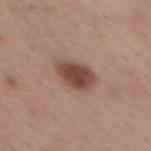Q: Was a biopsy performed?
A: total-body-photography surveillance lesion; no biopsy
Q: How large is the lesion?
A: about 4.5 mm
Q: Patient demographics?
A: female, about 50 years old
Q: What kind of image is this?
A: ~15 mm crop, total-body skin-cancer survey
Q: Automated lesion metrics?
A: a lesion area of about 9.5 mm², an eccentricity of roughly 0.8, and a symmetry-axis asymmetry near 0.25; a lesion color around L≈46 a*≈21 b*≈25 in CIELAB, a lesion–skin lightness drop of about 14, and a normalized lesion–skin contrast near 10.5
Q: Where on the body is the lesion?
A: the chest